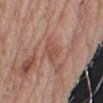Q: Is there a histopathology result?
A: imaged on a skin check; not biopsied
Q: Automated lesion metrics?
A: about 7 CIELAB-L* units darker than the surrounding skin and a normalized border contrast of about 5.5
Q: What is the anatomic site?
A: the right upper arm
Q: Lesion size?
A: about 3 mm
Q: What lighting was used for the tile?
A: white-light illumination
Q: Patient demographics?
A: male, in their mid-70s
Q: What is the imaging modality?
A: ~15 mm tile from a whole-body skin photo